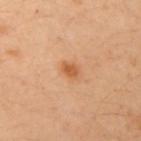Findings:
– subject · female, approximately 40 years of age
– diameter · ~2 mm (longest diameter)
– image source · ~15 mm tile from a whole-body skin photo
– image-analysis metrics · an outline eccentricity of about 0.55 (0 = round, 1 = elongated); a mean CIELAB color near L≈58 a*≈26 b*≈41, roughly 10 lightness units darker than nearby skin, and a normalized lesion–skin contrast near 7.5; border irregularity of about 2 on a 0–10 scale, a color-variation rating of about 2/10, and a peripheral color-asymmetry measure near 0.5; a classifier nevus-likeness of about 85/100
– body site · the right upper arm